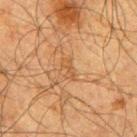notes: total-body-photography surveillance lesion; no biopsy | illumination: cross-polarized | image: ~15 mm crop, total-body skin-cancer survey | diameter: about 2.5 mm | subject: male, aged around 65 | body site: the right upper arm.